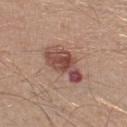Recorded during total-body skin imaging; not selected for excision or biopsy. Cropped from a whole-body photographic skin survey; the tile spans about 15 mm. A male patient, aged 63–67. Located on the left lower leg. Imaged with white-light lighting.